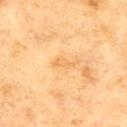biopsy status: no biopsy performed (imaged during a skin exam)
lesion size: ≈2.5 mm
tile lighting: cross-polarized illumination
anatomic site: the upper back
subject: male, aged around 70
acquisition: ~15 mm crop, total-body skin-cancer survey